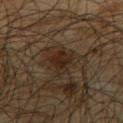Clinical impression:
The lesion was tiled from a total-body skin photograph and was not biopsied.
Image and clinical context:
The patient is a male approximately 65 years of age. Imaged with cross-polarized lighting. From the upper back. A region of skin cropped from a whole-body photographic capture, roughly 15 mm wide.